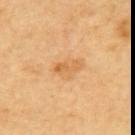Findings:
- workup · catalogued during a skin exam; not biopsied
- image-analysis metrics · an area of roughly 3 mm², a shape eccentricity near 0.95, and a shape-asymmetry score of about 0.45 (0 = symmetric); a border-irregularity index near 6/10 and a color-variation rating of about 0/10; an automated nevus-likeness rating near 5 out of 100 and lesion-presence confidence of about 100/100
- body site · the upper back
- image source · total-body-photography crop, ~15 mm field of view
- patient · female, aged 58 to 62